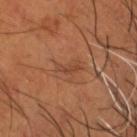Background: Automated tile analysis of the lesion measured border irregularity of about 6 on a 0–10 scale, a color-variation rating of about 0/10, and a peripheral color-asymmetry measure near 0. And it measured an automated nevus-likeness rating near 0 out of 100. A male patient aged approximately 55. Located on the head or neck. Captured under cross-polarized illumination. Cropped from a whole-body photographic skin survey; the tile spans about 15 mm. About 3 mm across.